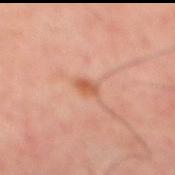Q: Was a biopsy performed?
A: imaged on a skin check; not biopsied
Q: Patient demographics?
A: male, aged 48 to 52
Q: How was the tile lit?
A: cross-polarized illumination
Q: What did automated image analysis measure?
A: a footprint of about 2.5 mm² and an outline eccentricity of about 0.75 (0 = round, 1 = elongated); a lesion color around L≈57 a*≈27 b*≈35 in CIELAB, roughly 10 lightness units darker than nearby skin, and a normalized lesion–skin contrast near 7.5; a color-variation rating of about 2/10 and a peripheral color-asymmetry measure near 0.5
Q: How was this image acquired?
A: 15 mm crop, total-body photography
Q: How large is the lesion?
A: about 2.5 mm
Q: Lesion location?
A: the mid back